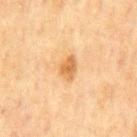Captured during whole-body skin photography for melanoma surveillance; the lesion was not biopsied.
The recorded lesion diameter is about 3 mm.
A 15 mm close-up tile from a total-body photography series done for melanoma screening.
The patient is a male approximately 70 years of age.
The tile uses cross-polarized illumination.
On the mid back.